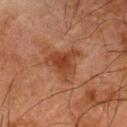Q: Was this lesion biopsied?
A: catalogued during a skin exam; not biopsied
Q: What lighting was used for the tile?
A: cross-polarized
Q: What is the imaging modality?
A: ~15 mm crop, total-body skin-cancer survey
Q: Automated lesion metrics?
A: a lesion color around L≈33 a*≈21 b*≈28 in CIELAB and a normalized lesion–skin contrast near 7.5; a nevus-likeness score of about 10/100 and a lesion-detection confidence of about 100/100
Q: Where on the body is the lesion?
A: the left thigh
Q: What are the patient's age and sex?
A: male, about 80 years old